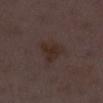Assessment:
Recorded during total-body skin imaging; not selected for excision or biopsy.
Acquisition and patient details:
Captured under white-light illumination. The total-body-photography lesion software estimated a classifier nevus-likeness of about 10/100 and a lesion-detection confidence of about 100/100. The subject is a female roughly 30 years of age. Cropped from a whole-body photographic skin survey; the tile spans about 15 mm. On the left lower leg.